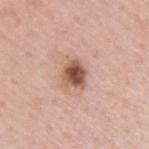Q: What is the lesion's diameter?
A: ~3.5 mm (longest diameter)
Q: Automated lesion metrics?
A: a lesion area of about 7.5 mm², an outline eccentricity of about 0.45 (0 = round, 1 = elongated), and a shape-asymmetry score of about 0.25 (0 = symmetric); a border-irregularity rating of about 2.5/10, a color-variation rating of about 7.5/10, and a peripheral color-asymmetry measure near 2.5; a nevus-likeness score of about 85/100
Q: Who is the patient?
A: male, in their 60s
Q: Illumination type?
A: white-light
Q: What is the imaging modality?
A: 15 mm crop, total-body photography
Q: What is the anatomic site?
A: the upper back